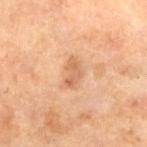Clinical impression:
Recorded during total-body skin imaging; not selected for excision or biopsy.
Context:
Captured under cross-polarized illumination. A female patient approximately 70 years of age. Automated image analysis of the tile measured an area of roughly 4.5 mm², an eccentricity of roughly 0.8, and a shape-asymmetry score of about 0.35 (0 = symmetric). The analysis additionally found an average lesion color of about L≈62 a*≈23 b*≈36 (CIELAB), a lesion–skin lightness drop of about 9, and a normalized lesion–skin contrast near 6. A region of skin cropped from a whole-body photographic capture, roughly 15 mm wide. Located on the left lower leg. Approximately 3 mm at its widest.